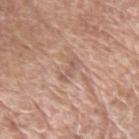illumination: white-light illumination | automated lesion analysis: a lesion area of about 2.5 mm²; a lesion color around L≈57 a*≈19 b*≈27 in CIELAB, a lesion–skin lightness drop of about 8, and a normalized border contrast of about 5.5; a nevus-likeness score of about 0/100 | imaging modality: ~15 mm crop, total-body skin-cancer survey | patient: male, in their 60s | location: the left upper arm | size: ≈3 mm.